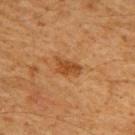The lesion was photographed on a routine skin check and not biopsied; there is no pathology result. From the upper back. This image is a 15 mm lesion crop taken from a total-body photograph. A male patient aged 58–62. Automated tile analysis of the lesion measured an area of roughly 5.5 mm², a shape eccentricity near 0.8, and a shape-asymmetry score of about 0.35 (0 = symmetric). The software also gave a mean CIELAB color near L≈39 a*≈21 b*≈35 and a normalized lesion–skin contrast near 7.5. The analysis additionally found border irregularity of about 3.5 on a 0–10 scale, a color-variation rating of about 2/10, and peripheral color asymmetry of about 0.5. Imaged with cross-polarized lighting.